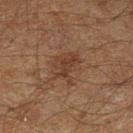Notes:
* notes — catalogued during a skin exam; not biopsied
* image source — 15 mm crop, total-body photography
* site — the left lower leg
* patient — male, aged around 60